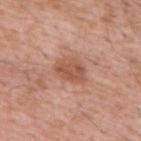Imaged during a routine full-body skin examination; the lesion was not biopsied and no histopathology is available.
On the chest.
Automated tile analysis of the lesion measured an area of roughly 8.5 mm², an outline eccentricity of about 0.7 (0 = round, 1 = elongated), and a shape-asymmetry score of about 0.2 (0 = symmetric). The software also gave a color-variation rating of about 3.5/10 and peripheral color asymmetry of about 1.
Longest diameter approximately 4 mm.
The tile uses white-light illumination.
A male subject, aged 58–62.
A close-up tile cropped from a whole-body skin photograph, about 15 mm across.